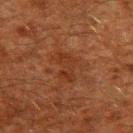Findings:
– biopsy status — no biopsy performed (imaged during a skin exam)
– patient — male, aged around 60
– location — the upper back
– image source — ~15 mm tile from a whole-body skin photo